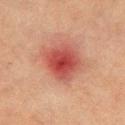Q: Was a biopsy performed?
A: total-body-photography surveillance lesion; no biopsy
Q: Who is the patient?
A: female, roughly 70 years of age
Q: Where on the body is the lesion?
A: the leg
Q: What is the lesion's diameter?
A: ~4.5 mm (longest diameter)
Q: How was this image acquired?
A: ~15 mm tile from a whole-body skin photo
Q: What did automated image analysis measure?
A: a mean CIELAB color near L≈44 a*≈31 b*≈27, roughly 12 lightness units darker than nearby skin, and a normalized lesion–skin contrast near 9; a classifier nevus-likeness of about 0/100 and lesion-presence confidence of about 100/100
Q: Illumination type?
A: cross-polarized illumination